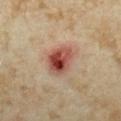Findings:
* workup: no biopsy performed (imaged during a skin exam)
* size: ≈4 mm
* image source: ~15 mm crop, total-body skin-cancer survey
* location: the left forearm
* subject: female, aged 33–37
* illumination: cross-polarized
* image-analysis metrics: an average lesion color of about L≈46 a*≈26 b*≈27 (CIELAB), about 15 CIELAB-L* units darker than the surrounding skin, and a lesion-to-skin contrast of about 11 (normalized; higher = more distinct)